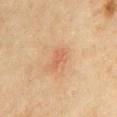notes: no biopsy performed (imaged during a skin exam) | lighting: cross-polarized illumination | patient: male, in their 70s | anatomic site: the chest | lesion size: ~2.5 mm (longest diameter) | image source: ~15 mm tile from a whole-body skin photo.